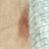• workup · imaged on a skin check; not biopsied
• tile lighting · cross-polarized illumination
• subject · female, aged around 40
• image source · ~15 mm tile from a whole-body skin photo
• diameter · ≈11 mm
• automated lesion analysis · a lesion area of about 32 mm², an outline eccentricity of about 0.9 (0 = round, 1 = elongated), and a shape-asymmetry score of about 0.25 (0 = symmetric)
• body site · the lower back